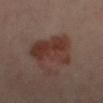Captured during whole-body skin photography for melanoma surveillance; the lesion was not biopsied. This is a cross-polarized tile. A female subject in their mid-60s. From the left leg. Automated tile analysis of the lesion measured a lesion area of about 20 mm², an eccentricity of roughly 0.65, and a shape-asymmetry score of about 0.3 (0 = symmetric). And it measured a mean CIELAB color near L≈33 a*≈19 b*≈21, roughly 8 lightness units darker than nearby skin, and a lesion-to-skin contrast of about 8.5 (normalized; higher = more distinct). A close-up tile cropped from a whole-body skin photograph, about 15 mm across. The recorded lesion diameter is about 6 mm.